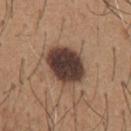biopsy status = catalogued during a skin exam; not biopsied.